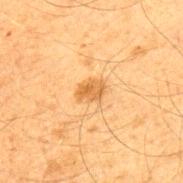Imaged during a routine full-body skin examination; the lesion was not biopsied and no histopathology is available. From the upper back. This is a cross-polarized tile. About 3 mm across. Cropped from a whole-body photographic skin survey; the tile spans about 15 mm. A male subject in their mid-60s.